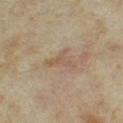notes=catalogued during a skin exam; not biopsied
site=the left thigh
diameter=about 3.5 mm
tile lighting=cross-polarized
image source=~15 mm crop, total-body skin-cancer survey
patient=female, approximately 35 years of age